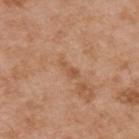Q: Was a biopsy performed?
A: no biopsy performed (imaged during a skin exam)
Q: Who is the patient?
A: male, about 55 years old
Q: What is the lesion's diameter?
A: about 3 mm
Q: How was this image acquired?
A: 15 mm crop, total-body photography
Q: Illumination type?
A: white-light
Q: What is the anatomic site?
A: the upper back